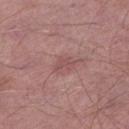This lesion was catalogued during total-body skin photography and was not selected for biopsy. From the left lower leg. A male patient in their mid-50s. A roughly 15 mm field-of-view crop from a total-body skin photograph.